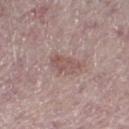<lesion>
<biopsy_status>not biopsied; imaged during a skin examination</biopsy_status>
<patient>
  <sex>male</sex>
  <age_approx>75</age_approx>
</patient>
<lighting>white-light</lighting>
<site>right thigh</site>
<image>
  <source>total-body photography crop</source>
  <field_of_view_mm>15</field_of_view_mm>
</image>
<lesion_size>
  <long_diameter_mm_approx>3.5</long_diameter_mm_approx>
</lesion_size>
</lesion>